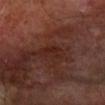Case summary:
- workup — imaged on a skin check; not biopsied
- lighting — cross-polarized illumination
- image source — 15 mm crop, total-body photography
- subject — male, in their 70s
- body site — the right forearm